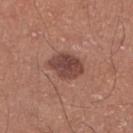Assessment:
The lesion was photographed on a routine skin check and not biopsied; there is no pathology result.
Background:
The lesion's longest dimension is about 4 mm. The subject is a male aged approximately 70. The lesion is on the right lower leg. A close-up tile cropped from a whole-body skin photograph, about 15 mm across.